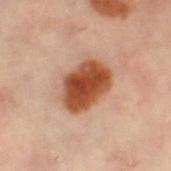The lesion was tiled from a total-body skin photograph and was not biopsied. The recorded lesion diameter is about 5.5 mm. A female subject about 60 years old. Cropped from a total-body skin-imaging series; the visible field is about 15 mm. Automated image analysis of the tile measured border irregularity of about 1.5 on a 0–10 scale and radial color variation of about 1.5. The lesion is located on the leg. The tile uses cross-polarized illumination.